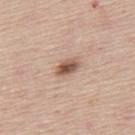Assessment:
The lesion was tiled from a total-body skin photograph and was not biopsied.
Image and clinical context:
A male subject, aged approximately 45. The lesion is on the mid back. This is a white-light tile. A 15 mm close-up tile from a total-body photography series done for melanoma screening. The recorded lesion diameter is about 3 mm.